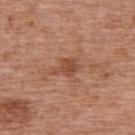Clinical impression: Imaged during a routine full-body skin examination; the lesion was not biopsied and no histopathology is available. Image and clinical context: Captured under white-light illumination. A 15 mm crop from a total-body photograph taken for skin-cancer surveillance. A female subject, aged 38–42. The recorded lesion diameter is about 3 mm. The lesion is located on the back.